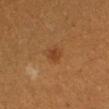This lesion was catalogued during total-body skin photography and was not selected for biopsy. The lesion is on the left forearm. Captured under cross-polarized illumination. Automated image analysis of the tile measured a lesion area of about 3 mm² and a shape eccentricity near 0.55. And it measured an average lesion color of about L≈38 a*≈21 b*≈34 (CIELAB) and a lesion–skin lightness drop of about 7. The analysis additionally found a classifier nevus-likeness of about 90/100 and a lesion-detection confidence of about 100/100. A female subject aged 28–32. A close-up tile cropped from a whole-body skin photograph, about 15 mm across. Longest diameter approximately 2 mm.